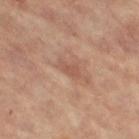Q: How was this image acquired?
A: 15 mm crop, total-body photography
Q: What is the lesion's diameter?
A: about 2.5 mm
Q: Who is the patient?
A: female, aged approximately 65
Q: What is the anatomic site?
A: the left leg
Q: Illumination type?
A: cross-polarized illumination